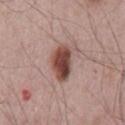This lesion was catalogued during total-body skin photography and was not selected for biopsy. A male patient roughly 65 years of age. The lesion is located on the mid back. Cropped from a whole-body photographic skin survey; the tile spans about 15 mm.